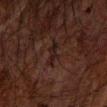Imaged during a routine full-body skin examination; the lesion was not biopsied and no histopathology is available.